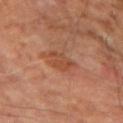No biopsy was performed on this lesion — it was imaged during a full skin examination and was not determined to be concerning. Located on the left upper arm. About 3 mm across. This is a cross-polarized tile. The subject is a male aged 63 to 67. This image is a 15 mm lesion crop taken from a total-body photograph. Automated image analysis of the tile measured a lesion color around L≈45 a*≈24 b*≈33 in CIELAB, roughly 7 lightness units darker than nearby skin, and a lesion-to-skin contrast of about 6 (normalized; higher = more distinct). And it measured a border-irregularity rating of about 3.5/10, a within-lesion color-variation index near 2/10, and radial color variation of about 1.